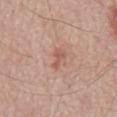Captured during whole-body skin photography for melanoma surveillance; the lesion was not biopsied. The total-body-photography lesion software estimated a lesion area of about 3 mm² and an outline eccentricity of about 0.85 (0 = round, 1 = elongated). And it measured a mean CIELAB color near L≈56 a*≈23 b*≈28. A lesion tile, about 15 mm wide, cut from a 3D total-body photograph. The subject is a male about 70 years old. The recorded lesion diameter is about 2.5 mm. This is a white-light tile. From the back.